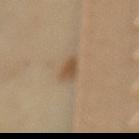No biopsy was performed on this lesion — it was imaged during a full skin examination and was not determined to be concerning. A female patient approximately 65 years of age. On the lower back. The tile uses cross-polarized illumination. A 15 mm close-up extracted from a 3D total-body photography capture.